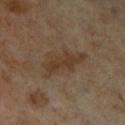<record>
  <biopsy_status>not biopsied; imaged during a skin examination</biopsy_status>
  <patient>
    <sex>female</sex>
    <age_approx>65</age_approx>
  </patient>
  <lighting>cross-polarized</lighting>
  <lesion_size>
    <long_diameter_mm_approx>6.0</long_diameter_mm_approx>
  </lesion_size>
  <site>left leg</site>
  <image>
    <source>total-body photography crop</source>
    <field_of_view_mm>15</field_of_view_mm>
  </image>
  <automated_metrics>
    <cielab_L>36</cielab_L>
    <cielab_a>14</cielab_a>
    <cielab_b>26</cielab_b>
    <vs_skin_darker_L>7.0</vs_skin_darker_L>
    <vs_skin_contrast_norm>7.0</vs_skin_contrast_norm>
    <border_irregularity_0_10>4.5</border_irregularity_0_10>
    <color_variation_0_10>2.5</color_variation_0_10>
    <peripheral_color_asymmetry>1.0</peripheral_color_asymmetry>
  </automated_metrics>
</record>